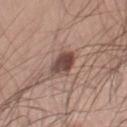Assessment: No biopsy was performed on this lesion — it was imaged during a full skin examination and was not determined to be concerning. Clinical summary: A 15 mm close-up tile from a total-body photography series done for melanoma screening. A male subject, approximately 30 years of age. From the right thigh.